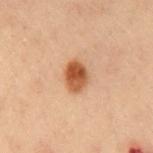follow-up = total-body-photography surveillance lesion; no biopsy
patient = male, in their mid-50s
site = the chest
tile lighting = cross-polarized illumination
image = ~15 mm crop, total-body skin-cancer survey
lesion size = about 3.5 mm